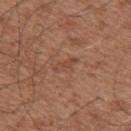Notes:
– workup · imaged on a skin check; not biopsied
– subject · male, aged 48 to 52
– site · the left upper arm
– image source · total-body-photography crop, ~15 mm field of view